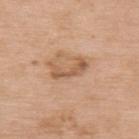follow-up: imaged on a skin check; not biopsied | location: the upper back | patient: female, aged around 75 | imaging modality: 15 mm crop, total-body photography.